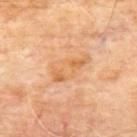Recorded during total-body skin imaging; not selected for excision or biopsy. Longest diameter approximately 5 mm. A male patient aged 63 to 67. A 15 mm close-up tile from a total-body photography series done for melanoma screening. Captured under cross-polarized illumination. The lesion is located on the upper back.